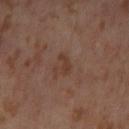Case summary:
• image source: total-body-photography crop, ~15 mm field of view
• patient: female, in their mid-50s
• lesion size: ≈3 mm
• TBP lesion metrics: a symmetry-axis asymmetry near 0.5; border irregularity of about 5 on a 0–10 scale, a color-variation rating of about 0/10, and radial color variation of about 0; a nevus-likeness score of about 0/100 and lesion-presence confidence of about 100/100
• anatomic site: the leg
• lighting: cross-polarized